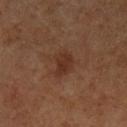Located on the left lower leg. An algorithmic analysis of the crop reported a border-irregularity rating of about 3/10, a within-lesion color-variation index near 1.5/10, and a peripheral color-asymmetry measure near 0.5. A region of skin cropped from a whole-body photographic capture, roughly 15 mm wide. The subject is a male aged 58–62. The recorded lesion diameter is about 3 mm.